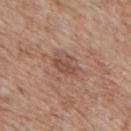The lesion was photographed on a routine skin check and not biopsied; there is no pathology result.
The patient is a male roughly 70 years of age.
About 3 mm across.
This image is a 15 mm lesion crop taken from a total-body photograph.
Imaged with white-light lighting.
From the mid back.